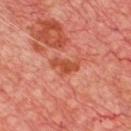No biopsy was performed on this lesion — it was imaged during a full skin examination and was not determined to be concerning.